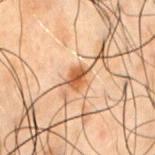<tbp_lesion>
<biopsy_status>not biopsied; imaged during a skin examination</biopsy_status>
<lighting>cross-polarized</lighting>
<site>chest</site>
<image>
  <source>total-body photography crop</source>
  <field_of_view_mm>15</field_of_view_mm>
</image>
<lesion_size>
  <long_diameter_mm_approx>2.5</long_diameter_mm_approx>
</lesion_size>
<patient>
  <sex>male</sex>
  <age_approx>50</age_approx>
</patient>
</tbp_lesion>